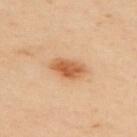Recorded during total-body skin imaging; not selected for excision or biopsy. A 15 mm close-up extracted from a 3D total-body photography capture. On the upper back. A male patient aged 63–67. The lesion's longest dimension is about 4 mm. This is a cross-polarized tile. Automated image analysis of the tile measured a mean CIELAB color near L≈59 a*≈25 b*≈40, about 13 CIELAB-L* units darker than the surrounding skin, and a lesion-to-skin contrast of about 9 (normalized; higher = more distinct). It also reported a within-lesion color-variation index near 4/10 and radial color variation of about 1.